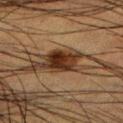The lesion was tiled from a total-body skin photograph and was not biopsied. The subject is a male approximately 50 years of age. A close-up tile cropped from a whole-body skin photograph, about 15 mm across. Located on the leg.